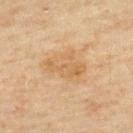• biopsy status — no biopsy performed (imaged during a skin exam)
• acquisition — ~15 mm tile from a whole-body skin photo
• subject — male, in their mid-40s
• size — about 5 mm
• lighting — cross-polarized
• body site — the upper back